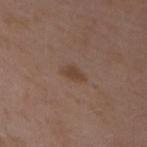* imaging modality · 15 mm crop, total-body photography
* patient · female, aged 28–32
* location · the left upper arm